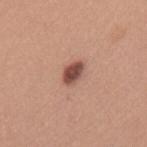Q: Was a biopsy performed?
A: total-body-photography surveillance lesion; no biopsy
Q: Where on the body is the lesion?
A: the upper back
Q: What is the imaging modality?
A: ~15 mm crop, total-body skin-cancer survey
Q: What are the patient's age and sex?
A: female, aged approximately 35